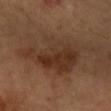Q: Was a biopsy performed?
A: no biopsy performed (imaged during a skin exam)
Q: Patient demographics?
A: female, aged approximately 60
Q: Automated lesion metrics?
A: a footprint of about 26 mm² and a symmetry-axis asymmetry near 0.4; an average lesion color of about L≈34 a*≈19 b*≈28 (CIELAB), a lesion–skin lightness drop of about 8, and a normalized border contrast of about 8; a border-irregularity rating of about 5.5/10, a within-lesion color-variation index near 5/10, and a peripheral color-asymmetry measure near 1.5; an automated nevus-likeness rating near 40 out of 100 and lesion-presence confidence of about 100/100
Q: How was this image acquired?
A: total-body-photography crop, ~15 mm field of view
Q: What lighting was used for the tile?
A: cross-polarized
Q: Where on the body is the lesion?
A: the arm